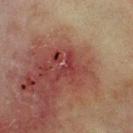<tbp_lesion>
<image>
  <source>total-body photography crop</source>
  <field_of_view_mm>15</field_of_view_mm>
</image>
<patient>
  <sex>male</sex>
  <age_approx>70</age_approx>
</patient>
<site>chest</site>
<diagnosis>
  <histopathology>superficial basal cell carcinoma</histopathology>
  <malignancy>malignant</malignancy>
  <taxonomic_path>Malignant; Malignant adnexal epithelial proliferations - Follicular; Basal cell carcinoma; Basal cell carcinoma, Superficial</taxonomic_path>
</diagnosis>
</tbp_lesion>